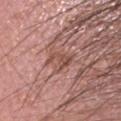An algorithmic analysis of the crop reported roughly 8 lightness units darker than nearby skin and a normalized lesion–skin contrast near 6. It also reported a color-variation rating of about 2/10 and a peripheral color-asymmetry measure near 0.5. The analysis additionally found an automated nevus-likeness rating near 0 out of 100 and a lesion-detection confidence of about 100/100.
A 15 mm crop from a total-body photograph taken for skin-cancer surveillance.
Measured at roughly 3 mm in maximum diameter.
Located on the head or neck.
A male subject, in their 60s.
Imaged with white-light lighting.